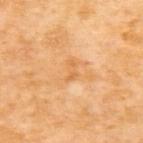Clinical impression:
The lesion was tiled from a total-body skin photograph and was not biopsied.
Clinical summary:
Measured at roughly 2.5 mm in maximum diameter. The lesion is on the upper back. The patient is a female in their mid- to late 50s. A close-up tile cropped from a whole-body skin photograph, about 15 mm across. Captured under cross-polarized illumination.